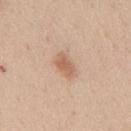This lesion was catalogued during total-body skin photography and was not selected for biopsy. The subject is a female aged 38 to 42. Automated tile analysis of the lesion measured an area of roughly 5 mm², an eccentricity of roughly 0.8, and a shape-asymmetry score of about 0.2 (0 = symmetric). And it measured border irregularity of about 2 on a 0–10 scale, a color-variation rating of about 2/10, and a peripheral color-asymmetry measure near 0.5. The lesion is on the mid back. A close-up tile cropped from a whole-body skin photograph, about 15 mm across. About 3 mm across. Imaged with white-light lighting.